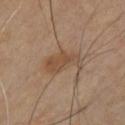Q: Was this lesion biopsied?
A: imaged on a skin check; not biopsied
Q: Patient demographics?
A: male, aged around 45
Q: Lesion location?
A: the chest
Q: How was this image acquired?
A: total-body-photography crop, ~15 mm field of view
Q: What lighting was used for the tile?
A: cross-polarized illumination
Q: Lesion size?
A: ~4.5 mm (longest diameter)